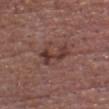<tbp_lesion>
<biopsy_status>not biopsied; imaged during a skin examination</biopsy_status>
<site>head or neck</site>
<patient>
  <sex>male</sex>
  <age_approx>80</age_approx>
</patient>
<lesion_size>
  <long_diameter_mm_approx>4.0</long_diameter_mm_approx>
</lesion_size>
<image>
  <source>total-body photography crop</source>
  <field_of_view_mm>15</field_of_view_mm>
</image>
<automated_metrics>
  <cielab_L>37</cielab_L>
  <cielab_a>21</cielab_a>
  <cielab_b>22</cielab_b>
  <vs_skin_darker_L>9.0</vs_skin_darker_L>
  <nevus_likeness_0_100>25</nevus_likeness_0_100>
</automated_metrics>
</tbp_lesion>